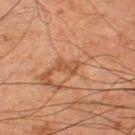notes: imaged on a skin check; not biopsied
TBP lesion metrics: a shape eccentricity near 0.85 and a symmetry-axis asymmetry near 0.4; a border-irregularity index near 4.5/10, internal color variation of about 1 on a 0–10 scale, and a peripheral color-asymmetry measure near 0; a nevus-likeness score of about 0/100 and a detector confidence of about 95 out of 100 that the crop contains a lesion
lighting: cross-polarized illumination
subject: male, roughly 80 years of age
body site: the left thigh
acquisition: ~15 mm tile from a whole-body skin photo